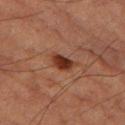{"biopsy_status": "not biopsied; imaged during a skin examination", "image": {"source": "total-body photography crop", "field_of_view_mm": 15}, "automated_metrics": {"cielab_L": 30, "cielab_a": 22, "cielab_b": 27, "vs_skin_darker_L": 12.0, "vs_skin_contrast_norm": 11.0, "color_variation_0_10": 3.5, "peripheral_color_asymmetry": 1.0, "nevus_likeness_0_100": 95, "lesion_detection_confidence_0_100": 100}, "patient": {"sex": "male", "age_approx": "85+"}, "lesion_size": {"long_diameter_mm_approx": 3.0}, "site": "left thigh", "lighting": "cross-polarized"}